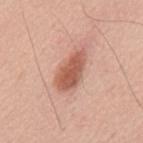No biopsy was performed on this lesion — it was imaged during a full skin examination and was not determined to be concerning. A male patient, aged 58 to 62. A region of skin cropped from a whole-body photographic capture, roughly 15 mm wide. Located on the mid back.